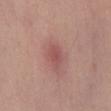workup = total-body-photography surveillance lesion; no biopsy | subject = male, aged 53–57 | image = 15 mm crop, total-body photography | site = the chest | automated lesion analysis = a lesion area of about 4 mm², an eccentricity of roughly 0.75, and two-axis asymmetry of about 0.2; an automated nevus-likeness rating near 0 out of 100 and a lesion-detection confidence of about 100/100 | tile lighting = white-light illumination.